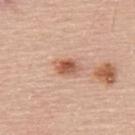{"site": "back", "lighting": "white-light", "automated_metrics": {"area_mm2_approx": 5.0, "eccentricity": 0.75, "shape_asymmetry": 0.25, "cielab_L": 58, "cielab_a": 24, "cielab_b": 31, "border_irregularity_0_10": 2.5, "color_variation_0_10": 6.0}, "image": {"source": "total-body photography crop", "field_of_view_mm": 15}, "lesion_size": {"long_diameter_mm_approx": 3.0}, "patient": {"sex": "male", "age_approx": 60}}